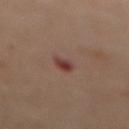Notes:
- image-analysis metrics — an area of roughly 3 mm²; a lesion color around L≈39 a*≈23 b*≈23 in CIELAB, a lesion–skin lightness drop of about 11, and a normalized lesion–skin contrast near 9; border irregularity of about 2 on a 0–10 scale, a within-lesion color-variation index near 3.5/10, and radial color variation of about 1; a nevus-likeness score of about 40/100 and a detector confidence of about 100 out of 100 that the crop contains a lesion
- illumination — cross-polarized illumination
- patient — female, aged 53 to 57
- site — the abdomen
- image source — total-body-photography crop, ~15 mm field of view